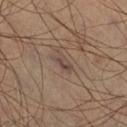  biopsy_status: not biopsied; imaged during a skin examination
  lighting: cross-polarized
  automated_metrics:
    area_mm2_approx: 3.5
    eccentricity: 0.9
    shape_asymmetry: 0.3
    cielab_L: 41
    cielab_a: 14
    cielab_b: 20
    vs_skin_darker_L: 7.0
    vs_skin_contrast_norm: 6.5
    nevus_likeness_0_100: 0
    lesion_detection_confidence_0_100: 70
  image:
    source: total-body photography crop
    field_of_view_mm: 15
  lesion_size:
    long_diameter_mm_approx: 3.0
  patient:
    sex: male
    age_approx: 45
  site: leg